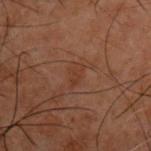• biopsy status · no biopsy performed (imaged during a skin exam)
• size · ≈2.5 mm
• location · the chest
• tile lighting · cross-polarized
• acquisition · ~15 mm tile from a whole-body skin photo
• image-analysis metrics · an area of roughly 3 mm², an outline eccentricity of about 0.85 (0 = round, 1 = elongated), and a shape-asymmetry score of about 0.35 (0 = symmetric); a lesion color around L≈27 a*≈17 b*≈24 in CIELAB, roughly 4 lightness units darker than nearby skin, and a lesion-to-skin contrast of about 5 (normalized; higher = more distinct); a border-irregularity rating of about 3.5/10, internal color variation of about 0.5 on a 0–10 scale, and radial color variation of about 0; a classifier nevus-likeness of about 0/100
• patient · male, aged 48 to 52